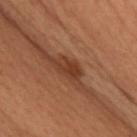Captured during whole-body skin photography for melanoma surveillance; the lesion was not biopsied.
The lesion is on the chest.
The subject is a female roughly 50 years of age.
A lesion tile, about 15 mm wide, cut from a 3D total-body photograph.